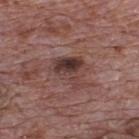No biopsy was performed on this lesion — it was imaged during a full skin examination and was not determined to be concerning. A male subject about 70 years old. Cropped from a total-body skin-imaging series; the visible field is about 15 mm. Imaged with white-light lighting. Located on the upper back. The recorded lesion diameter is about 4.5 mm.